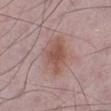Case summary:
– patient: male, about 50 years old
– anatomic site: the leg
– automated metrics: roughly 9 lightness units darker than nearby skin and a normalized lesion–skin contrast near 7; border irregularity of about 2.5 on a 0–10 scale, a color-variation rating of about 4/10, and peripheral color asymmetry of about 1; lesion-presence confidence of about 100/100
– acquisition: ~15 mm tile from a whole-body skin photo
– diameter: ~4.5 mm (longest diameter)